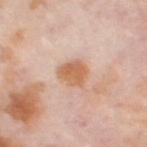workup = total-body-photography surveillance lesion; no biopsy
subject = female, aged 53 to 57
body site = the right thigh
lighting = cross-polarized
image source = ~15 mm tile from a whole-body skin photo